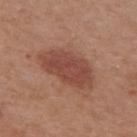workup — total-body-photography surveillance lesion; no biopsy
subject — female, in their 40s
acquisition — total-body-photography crop, ~15 mm field of view
site — the upper back